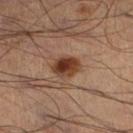{"biopsy_status": "not biopsied; imaged during a skin examination", "image": {"source": "total-body photography crop", "field_of_view_mm": 15}, "site": "left leg", "lesion_size": {"long_diameter_mm_approx": 3.5}, "lighting": "cross-polarized", "automated_metrics": {"area_mm2_approx": 6.5, "eccentricity": 0.65, "shape_asymmetry": 0.15, "cielab_L": 37, "cielab_a": 21, "cielab_b": 29, "vs_skin_darker_L": 14.0, "vs_skin_contrast_norm": 11.5}, "patient": {"sex": "male", "age_approx": 50}}